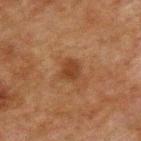follow-up=imaged on a skin check; not biopsied
anatomic site=the upper back
image source=total-body-photography crop, ~15 mm field of view
subject=male, roughly 60 years of age
automated lesion analysis=a lesion color around L≈31 a*≈18 b*≈28 in CIELAB, a lesion–skin lightness drop of about 7, and a normalized border contrast of about 7.5; border irregularity of about 2 on a 0–10 scale, internal color variation of about 1.5 on a 0–10 scale, and radial color variation of about 0.5; lesion-presence confidence of about 100/100
tile lighting=cross-polarized